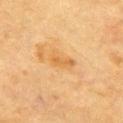follow-up — total-body-photography surveillance lesion; no biopsy | subject — male, in their mid- to late 80s or older | lesion diameter — ~3.5 mm (longest diameter) | image source — total-body-photography crop, ~15 mm field of view | TBP lesion metrics — a lesion color around L≈57 a*≈20 b*≈42 in CIELAB, about 7 CIELAB-L* units darker than the surrounding skin, and a lesion-to-skin contrast of about 6 (normalized; higher = more distinct); internal color variation of about 2 on a 0–10 scale and a peripheral color-asymmetry measure near 0.5; lesion-presence confidence of about 100/100 | anatomic site — the chest | lighting — cross-polarized illumination.